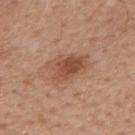follow-up: imaged on a skin check; not biopsied | imaging modality: ~15 mm crop, total-body skin-cancer survey | body site: the mid back | lesion size: ~4.5 mm (longest diameter) | image-analysis metrics: a shape-asymmetry score of about 0.3 (0 = symmetric) | lighting: white-light illumination | patient: male, aged 48–52.